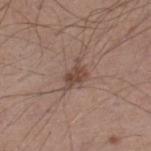Clinical impression: The lesion was tiled from a total-body skin photograph and was not biopsied. Clinical summary: The patient is a male aged 38 to 42. This is a white-light tile. A roughly 15 mm field-of-view crop from a total-body skin photograph. Longest diameter approximately 3.5 mm. The lesion is on the leg.